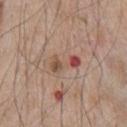  biopsy_status: not biopsied; imaged during a skin examination
  site: chest
  image:
    source: total-body photography crop
    field_of_view_mm: 15
  patient:
    sex: male
    age_approx: 65